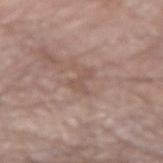Imaged during a routine full-body skin examination; the lesion was not biopsied and no histopathology is available.